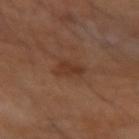notes: no biopsy performed (imaged during a skin exam) | acquisition: 15 mm crop, total-body photography | diameter: about 3 mm | automated metrics: a shape eccentricity near 0.75 and two-axis asymmetry of about 0.4; a mean CIELAB color near L≈33 a*≈20 b*≈28, a lesion–skin lightness drop of about 7, and a lesion-to-skin contrast of about 6.5 (normalized; higher = more distinct); a border-irregularity rating of about 4/10, a within-lesion color-variation index near 1.5/10, and peripheral color asymmetry of about 0.5 | subject: male, in their mid- to late 60s | illumination: cross-polarized illumination | body site: the left forearm.